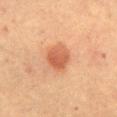size: about 3.5 mm
body site: the abdomen
imaging modality: total-body-photography crop, ~15 mm field of view
subject: female, aged approximately 60
tile lighting: cross-polarized illumination
automated metrics: about 10 CIELAB-L* units darker than the surrounding skin and a lesion-to-skin contrast of about 7 (normalized; higher = more distinct); a classifier nevus-likeness of about 100/100 and a lesion-detection confidence of about 100/100